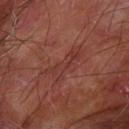biopsy status: total-body-photography surveillance lesion; no biopsy
anatomic site: the left forearm
diameter: ≈5 mm
tile lighting: cross-polarized
subject: male, roughly 70 years of age
acquisition: ~15 mm tile from a whole-body skin photo